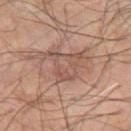Acquisition and patient details: This is a cross-polarized tile. Cropped from a total-body skin-imaging series; the visible field is about 15 mm. The lesion is on the left forearm. The recorded lesion diameter is about 4 mm. A male patient approximately 65 years of age.